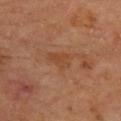This lesion was catalogued during total-body skin photography and was not selected for biopsy.
On the upper back.
A roughly 15 mm field-of-view crop from a total-body skin photograph.
A male patient, in their mid- to late 60s.